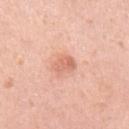The lesion was tiled from a total-body skin photograph and was not biopsied.
A female subject, aged around 30.
A 15 mm close-up extracted from a 3D total-body photography capture.
The lesion is located on the left upper arm.
Captured under white-light illumination.
An algorithmic analysis of the crop reported a lesion color around L≈67 a*≈26 b*≈32 in CIELAB, a lesion–skin lightness drop of about 10, and a normalized border contrast of about 6.
Approximately 3 mm at its widest.